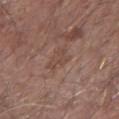notes: imaged on a skin check; not biopsied | lesion size: ~3 mm (longest diameter) | automated lesion analysis: a footprint of about 4 mm², an outline eccentricity of about 0.65 (0 = round, 1 = elongated), and a symmetry-axis asymmetry near 0.6; a border-irregularity rating of about 7/10 and radial color variation of about 0 | illumination: white-light illumination | subject: male, aged 63 to 67 | image: ~15 mm tile from a whole-body skin photo | anatomic site: the right forearm.